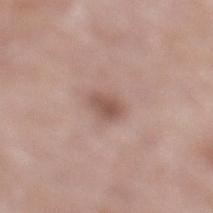  biopsy_status: not biopsied; imaged during a skin examination
  patient:
    sex: male
    age_approx: 50
  lesion_size:
    long_diameter_mm_approx: 2.5
  automated_metrics:
    area_mm2_approx: 3.5
    eccentricity: 0.8
    shape_asymmetry: 0.3
    nevus_likeness_0_100: 65
    lesion_detection_confidence_0_100: 100
  lighting: white-light
  image:
    source: total-body photography crop
    field_of_view_mm: 15
  site: left lower leg